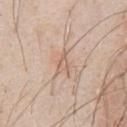Case summary:
- follow-up: catalogued during a skin exam; not biopsied
- image source: ~15 mm tile from a whole-body skin photo
- location: the chest
- subject: male, in their mid-40s